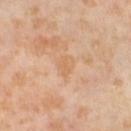Captured during whole-body skin photography for melanoma surveillance; the lesion was not biopsied.
A female patient aged 53–57.
The tile uses cross-polarized illumination.
The lesion-visualizer software estimated a border-irregularity index near 3/10 and radial color variation of about 0.5. The analysis additionally found a nevus-likeness score of about 0/100 and a lesion-detection confidence of about 100/100.
A 15 mm close-up tile from a total-body photography series done for melanoma screening.
The lesion's longest dimension is about 3 mm.
The lesion is located on the left thigh.